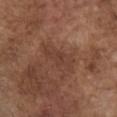Acquisition and patient details:
A region of skin cropped from a whole-body photographic capture, roughly 15 mm wide. Approximately 5 mm at its widest. On the chest. A male subject aged around 75.